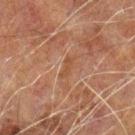Notes:
• follow-up — no biopsy performed (imaged during a skin exam)
• illumination — cross-polarized illumination
• diameter — ~2.5 mm (longest diameter)
• automated metrics — an area of roughly 2 mm², an eccentricity of roughly 0.95, and a shape-asymmetry score of about 0.4 (0 = symmetric); a border-irregularity rating of about 4.5/10 and radial color variation of about 0
• body site — the arm
• image — 15 mm crop, total-body photography
• subject — male, aged 58–62